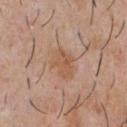The lesion was photographed on a routine skin check and not biopsied; there is no pathology result.
Longest diameter approximately 3.5 mm.
The lesion is on the chest.
A 15 mm close-up extracted from a 3D total-body photography capture.
The subject is a male aged around 30.
Imaged with white-light lighting.